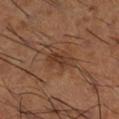This lesion was catalogued during total-body skin photography and was not selected for biopsy. A 15 mm crop from a total-body photograph taken for skin-cancer surveillance. On the right lower leg. Approximately 3.5 mm at its widest. A male subject, aged 63 to 67.